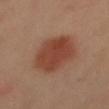The lesion was photographed on a routine skin check and not biopsied; there is no pathology result.
Imaged with cross-polarized lighting.
Approximately 6.5 mm at its widest.
This image is a 15 mm lesion crop taken from a total-body photograph.
A female patient.